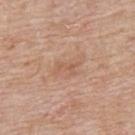patient = male, about 65 years old; image source = 15 mm crop, total-body photography; illumination = white-light; anatomic site = the upper back.